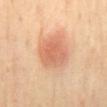Background: Automated tile analysis of the lesion measured a border-irregularity rating of about 1.5/10 and internal color variation of about 4 on a 0–10 scale. The lesion is on the back. A male patient, roughly 65 years of age. The tile uses cross-polarized illumination. This image is a 15 mm lesion crop taken from a total-body photograph.